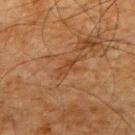image:
  source: total-body photography crop
  field_of_view_mm: 15
site: back
patient:
  sex: male
  age_approx: 65
lighting: cross-polarized